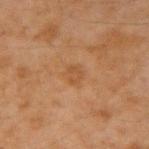biopsy status = imaged on a skin check; not biopsied
automated lesion analysis = internal color variation of about 2 on a 0–10 scale and radial color variation of about 0.5; a classifier nevus-likeness of about 0/100 and a lesion-detection confidence of about 100/100
subject = male, roughly 45 years of age
image = ~15 mm crop, total-body skin-cancer survey
body site = the arm